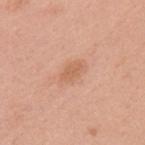Part of a total-body skin-imaging series; this lesion was reviewed on a skin check and was not flagged for biopsy.
The tile uses white-light illumination.
The lesion is on the upper back.
A 15 mm close-up tile from a total-body photography series done for melanoma screening.
A female subject, approximately 50 years of age.
Longest diameter approximately 3 mm.